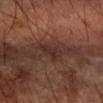Assessment:
No biopsy was performed on this lesion — it was imaged during a full skin examination and was not determined to be concerning.